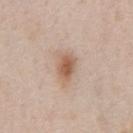– workup: total-body-photography surveillance lesion; no biopsy
– lighting: white-light illumination
– subject: male, in their 60s
– imaging modality: ~15 mm crop, total-body skin-cancer survey
– size: about 3.5 mm
– site: the chest